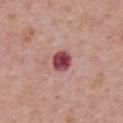This lesion was catalogued during total-body skin photography and was not selected for biopsy. Longest diameter approximately 2.5 mm. The patient is a female in their mid- to late 60s. On the upper back. A roughly 15 mm field-of-view crop from a total-body skin photograph. Imaged with white-light lighting.